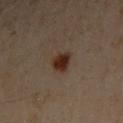This is a cross-polarized tile. From the left arm. This image is a 15 mm lesion crop taken from a total-body photograph. A male subject, in their 50s.